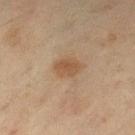  automated_metrics:
    area_mm2_approx: 5.0
    eccentricity: 0.7
    shape_asymmetry: 0.25
  site: left lower leg
  image:
    source: total-body photography crop
    field_of_view_mm: 15
  patient:
    sex: female
    age_approx: 55
  lighting: cross-polarized